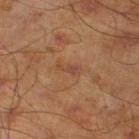This lesion was catalogued during total-body skin photography and was not selected for biopsy. The lesion is on the left lower leg. A roughly 15 mm field-of-view crop from a total-body skin photograph. The tile uses cross-polarized illumination. A male subject in their mid- to late 40s. Measured at roughly 3 mm in maximum diameter. Automated image analysis of the tile measured a lesion color around L≈45 a*≈22 b*≈31 in CIELAB, roughly 7 lightness units darker than nearby skin, and a normalized border contrast of about 5.5.